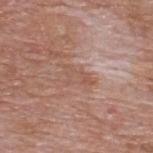{"biopsy_status": "not biopsied; imaged during a skin examination", "image": {"source": "total-body photography crop", "field_of_view_mm": 15}, "lighting": "white-light", "site": "upper back", "lesion_size": {"long_diameter_mm_approx": 3.5}, "patient": {"sex": "male", "age_approx": 60}}